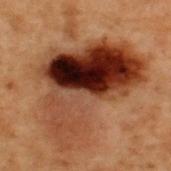<record>
<diagnosis>
  <histopathology>melanoma in situ</histopathology>
  <malignancy>malignant</malignancy>
  <taxonomic_path>Malignant, Malignant melanocytic proliferations (Melanoma), Melanoma in situ</taxonomic_path>
</diagnosis>
</record>